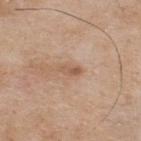{
  "biopsy_status": "not biopsied; imaged during a skin examination",
  "patient": {
    "sex": "male",
    "age_approx": 75
  },
  "image": {
    "source": "total-body photography crop",
    "field_of_view_mm": 15
  },
  "lesion_size": {
    "long_diameter_mm_approx": 2.5
  },
  "site": "upper back"
}